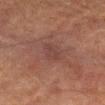Q: Is there a histopathology result?
A: no biopsy performed (imaged during a skin exam)
Q: How was this image acquired?
A: ~15 mm tile from a whole-body skin photo
Q: How was the tile lit?
A: cross-polarized illumination
Q: What is the lesion's diameter?
A: ~4 mm (longest diameter)
Q: Automated lesion metrics?
A: an area of roughly 5.5 mm², an outline eccentricity of about 0.9 (0 = round, 1 = elongated), and a shape-asymmetry score of about 0.2 (0 = symmetric); a lesion color around L≈33 a*≈18 b*≈19 in CIELAB, roughly 5 lightness units darker than nearby skin, and a lesion-to-skin contrast of about 4.5 (normalized; higher = more distinct); a classifier nevus-likeness of about 0/100 and a detector confidence of about 95 out of 100 that the crop contains a lesion
Q: Patient demographics?
A: male, aged 63 to 67
Q: What is the anatomic site?
A: the right forearm